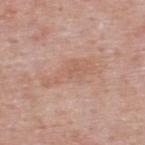A male subject aged approximately 55.
On the upper back.
A roughly 15 mm field-of-view crop from a total-body skin photograph.